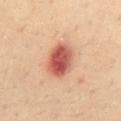Findings:
* notes: no biopsy performed (imaged during a skin exam)
* image-analysis metrics: a shape eccentricity near 0.65 and a shape-asymmetry score of about 0.1 (0 = symmetric); internal color variation of about 7.5 on a 0–10 scale and peripheral color asymmetry of about 2.5; a lesion-detection confidence of about 100/100
* site: the mid back
* lighting: cross-polarized illumination
* size: about 4.5 mm
* patient: male, aged 33–37
* image: ~15 mm crop, total-body skin-cancer survey